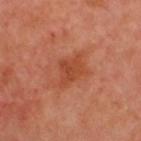<lesion>
  <biopsy_status>not biopsied; imaged during a skin examination</biopsy_status>
  <image>
    <source>total-body photography crop</source>
    <field_of_view_mm>15</field_of_view_mm>
  </image>
  <site>back</site>
  <patient>
    <sex>male</sex>
    <age_approx>50</age_approx>
  </patient>
</lesion>